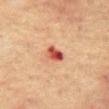follow-up = no biopsy performed (imaged during a skin exam); lighting = cross-polarized illumination; subject = male, aged around 65; image = ~15 mm tile from a whole-body skin photo; size = ~2.5 mm (longest diameter); TBP lesion metrics = a classifier nevus-likeness of about 0/100 and lesion-presence confidence of about 100/100; location = the abdomen.